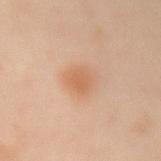Impression: Part of a total-body skin-imaging series; this lesion was reviewed on a skin check and was not flagged for biopsy. Acquisition and patient details: A female patient, aged 53–57. The lesion-visualizer software estimated a mean CIELAB color near L≈64 a*≈22 b*≈37. Captured under cross-polarized illumination. About 3 mm across. The lesion is on the left forearm. A region of skin cropped from a whole-body photographic capture, roughly 15 mm wide.